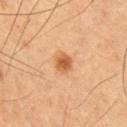Q: Was a biopsy performed?
A: catalogued during a skin exam; not biopsied
Q: What lighting was used for the tile?
A: cross-polarized
Q: Lesion size?
A: about 2.5 mm
Q: What are the patient's age and sex?
A: male, aged 58 to 62
Q: How was this image acquired?
A: 15 mm crop, total-body photography
Q: What is the anatomic site?
A: the left thigh